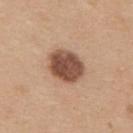follow-up: catalogued during a skin exam; not biopsied
patient: male, in their mid- to late 30s
site: the upper back
illumination: white-light
image source: ~15 mm tile from a whole-body skin photo
automated lesion analysis: a border-irregularity rating of about 1.5/10, internal color variation of about 4 on a 0–10 scale, and radial color variation of about 1.5; a classifier nevus-likeness of about 45/100 and a lesion-detection confidence of about 100/100
size: ≈4.5 mm